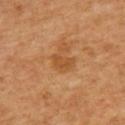No biopsy was performed on this lesion — it was imaged during a full skin examination and was not determined to be concerning.